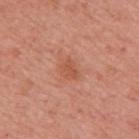{
  "biopsy_status": "not biopsied; imaged during a skin examination",
  "site": "arm",
  "automated_metrics": {
    "cielab_L": 54,
    "cielab_a": 27,
    "cielab_b": 33,
    "vs_skin_darker_L": 7.0,
    "vs_skin_contrast_norm": 5.5,
    "border_irregularity_0_10": 2.5,
    "color_variation_0_10": 2.0,
    "peripheral_color_asymmetry": 0.5,
    "nevus_likeness_0_100": 30,
    "lesion_detection_confidence_0_100": 100
  },
  "lesion_size": {
    "long_diameter_mm_approx": 2.5
  },
  "patient": {
    "sex": "female",
    "age_approx": 50
  },
  "image": {
    "source": "total-body photography crop",
    "field_of_view_mm": 15
  }
}